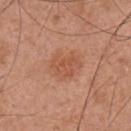This lesion was catalogued during total-body skin photography and was not selected for biopsy.
A male patient, aged 53–57.
The lesion-visualizer software estimated a footprint of about 8 mm², a shape eccentricity near 0.65, and a symmetry-axis asymmetry near 0.35. And it measured an average lesion color of about L≈54 a*≈25 b*≈33 (CIELAB) and a normalized border contrast of about 5.5. The software also gave border irregularity of about 3.5 on a 0–10 scale, a within-lesion color-variation index near 2.5/10, and radial color variation of about 1. And it measured an automated nevus-likeness rating near 15 out of 100 and a detector confidence of about 100 out of 100 that the crop contains a lesion.
This is a white-light tile.
This image is a 15 mm lesion crop taken from a total-body photograph.
Approximately 3.5 mm at its widest.
On the front of the torso.